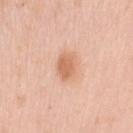Imaged during a routine full-body skin examination; the lesion was not biopsied and no histopathology is available. Located on the right upper arm. The subject is a female in their mid- to late 50s. Imaged with white-light lighting. A lesion tile, about 15 mm wide, cut from a 3D total-body photograph.